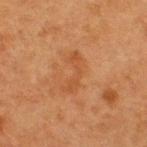workup = no biopsy performed (imaged during a skin exam)
size = about 4.5 mm
image source = ~15 mm tile from a whole-body skin photo
subject = female, aged approximately 40
lighting = cross-polarized
site = the upper back